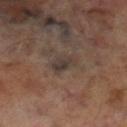Imaged during a routine full-body skin examination; the lesion was not biopsied and no histopathology is available. A 15 mm crop from a total-body photograph taken for skin-cancer surveillance. A male subject, aged approximately 70. On the right lower leg.